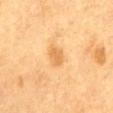biopsy status: imaged on a skin check; not biopsied
image-analysis metrics: an area of roughly 5 mm², an outline eccentricity of about 0.7 (0 = round, 1 = elongated), and a symmetry-axis asymmetry near 0.2; an average lesion color of about L≈60 a*≈20 b*≈42 (CIELAB) and a lesion-to-skin contrast of about 6 (normalized; higher = more distinct); a border-irregularity index near 2/10 and internal color variation of about 1.5 on a 0–10 scale; an automated nevus-likeness rating near 5 out of 100 and a detector confidence of about 100 out of 100 that the crop contains a lesion
location: the abdomen
lesion size: about 2.5 mm
acquisition: ~15 mm tile from a whole-body skin photo
subject: male, aged approximately 85 or older